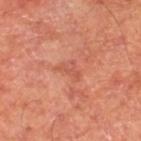No biopsy was performed on this lesion — it was imaged during a full skin examination and was not determined to be concerning. A region of skin cropped from a whole-body photographic capture, roughly 15 mm wide. Automated image analysis of the tile measured a footprint of about 3.5 mm², an eccentricity of roughly 0.8, and a symmetry-axis asymmetry near 0.55. And it measured a lesion color around L≈55 a*≈30 b*≈33 in CIELAB and about 7 CIELAB-L* units darker than the surrounding skin. It also reported an automated nevus-likeness rating near 0 out of 100. Captured under cross-polarized illumination. The lesion is on the right lower leg. The subject is a male aged 63 to 67. Approximately 3 mm at its widest.